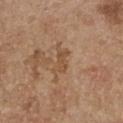The lesion was tiled from a total-body skin photograph and was not biopsied.
On the chest.
A roughly 15 mm field-of-view crop from a total-body skin photograph.
The tile uses white-light illumination.
The patient is a female aged 63 to 67.
The recorded lesion diameter is about 3.5 mm.